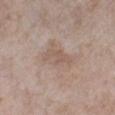Captured during whole-body skin photography for melanoma surveillance; the lesion was not biopsied. The lesion is located on the right lower leg. The subject is a female aged around 55. Cropped from a total-body skin-imaging series; the visible field is about 15 mm.